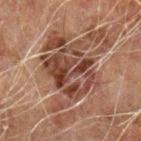This lesion was catalogued during total-body skin photography and was not selected for biopsy.
A male subject, roughly 60 years of age.
From the left forearm.
A 15 mm crop from a total-body photograph taken for skin-cancer surveillance.